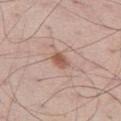<tbp_lesion>
<biopsy_status>not biopsied; imaged during a skin examination</biopsy_status>
<image>
  <source>total-body photography crop</source>
  <field_of_view_mm>15</field_of_view_mm>
</image>
<lighting>white-light</lighting>
<lesion_size>
  <long_diameter_mm_approx>2.5</long_diameter_mm_approx>
</lesion_size>
<site>left thigh</site>
<automated_metrics>
  <area_mm2_approx>3.5</area_mm2_approx>
  <eccentricity>0.75</eccentricity>
  <shape_asymmetry>0.2</shape_asymmetry>
  <cielab_L>56</cielab_L>
  <cielab_a>21</cielab_a>
  <cielab_b>28</cielab_b>
  <vs_skin_darker_L>11.0</vs_skin_darker_L>
  <vs_skin_contrast_norm>8.0</vs_skin_contrast_norm>
</automated_metrics>
<patient>
  <sex>male</sex>
  <age_approx>60</age_approx>
</patient>
</tbp_lesion>